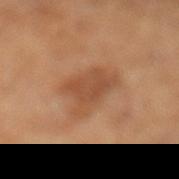{"biopsy_status": "not biopsied; imaged during a skin examination", "lesion_size": {"long_diameter_mm_approx": 5.5}, "image": {"source": "total-body photography crop", "field_of_view_mm": 15}, "site": "leg", "patient": {"sex": "male", "age_approx": 60}, "lighting": "cross-polarized"}